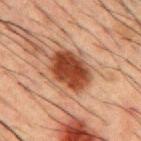<record>
  <biopsy_status>not biopsied; imaged during a skin examination</biopsy_status>
  <image>
    <source>total-body photography crop</source>
    <field_of_view_mm>15</field_of_view_mm>
  </image>
  <automated_metrics>
    <area_mm2_approx>15.0</area_mm2_approx>
    <eccentricity>0.7</eccentricity>
    <shape_asymmetry>0.1</shape_asymmetry>
    <border_irregularity_0_10>1.5</border_irregularity_0_10>
    <color_variation_0_10>4.0</color_variation_0_10>
    <peripheral_color_asymmetry>1.5</peripheral_color_asymmetry>
  </automated_metrics>
  <patient>
    <sex>male</sex>
    <age_approx>50</age_approx>
  </patient>
  <lesion_size>
    <long_diameter_mm_approx>5.0</long_diameter_mm_approx>
  </lesion_size>
  <site>mid back</site>
</record>